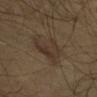illumination: cross-polarized illumination | patient: male, approximately 65 years of age | imaging modality: total-body-photography crop, ~15 mm field of view | automated lesion analysis: two-axis asymmetry of about 0.45; a lesion–skin lightness drop of about 6 and a lesion-to-skin contrast of about 6.5 (normalized; higher = more distinct); a nevus-likeness score of about 20/100 and lesion-presence confidence of about 95/100 | lesion size: about 4.5 mm | location: the front of the torso.